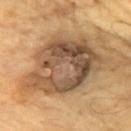The total-body-photography lesion software estimated a nevus-likeness score of about 10/100 and a detector confidence of about 35 out of 100 that the crop contains a lesion. A female subject. A roughly 15 mm field-of-view crop from a total-body skin photograph. Longest diameter approximately 9.5 mm. From the chest. Histopathologically confirmed as a seborrheic keratosis.